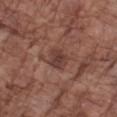Impression:
Recorded during total-body skin imaging; not selected for excision or biopsy.
Context:
The lesion is located on the left forearm. A 15 mm close-up tile from a total-body photography series done for melanoma screening. A male patient, roughly 75 years of age.